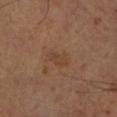Impression: Part of a total-body skin-imaging series; this lesion was reviewed on a skin check and was not flagged for biopsy. Clinical summary: The patient is a male approximately 55 years of age. The tile uses cross-polarized illumination. Automated image analysis of the tile measured an average lesion color of about L≈40 a*≈17 b*≈28 (CIELAB) and a lesion–skin lightness drop of about 5. It also reported internal color variation of about 3 on a 0–10 scale. Measured at roughly 3 mm in maximum diameter. A 15 mm close-up extracted from a 3D total-body photography capture. From the left lower leg.